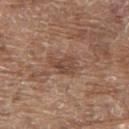| field | value |
|---|---|
| subject | male, aged 78–82 |
| anatomic site | the upper back |
| imaging modality | 15 mm crop, total-body photography |